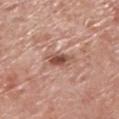Captured during whole-body skin photography for melanoma surveillance; the lesion was not biopsied.
A male patient about 70 years old.
The lesion is on the right lower leg.
About 4 mm across.
Captured under white-light illumination.
A 15 mm close-up tile from a total-body photography series done for melanoma screening.